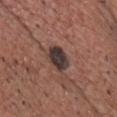Clinical summary: The lesion-visualizer software estimated a lesion color around L≈35 a*≈15 b*≈17 in CIELAB, about 14 CIELAB-L* units darker than the surrounding skin, and a normalized lesion–skin contrast near 13. The analysis additionally found an automated nevus-likeness rating near 10 out of 100. A male subject in their mid-60s. The lesion's longest dimension is about 3.5 mm. From the chest. A 15 mm close-up extracted from a 3D total-body photography capture.